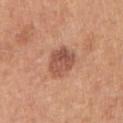Impression: Imaged during a routine full-body skin examination; the lesion was not biopsied and no histopathology is available. Background: Measured at roughly 4 mm in maximum diameter. A male patient in their 30s. A region of skin cropped from a whole-body photographic capture, roughly 15 mm wide. This is a white-light tile. The lesion is on the right upper arm.